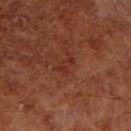{"biopsy_status": "not biopsied; imaged during a skin examination", "lesion_size": {"long_diameter_mm_approx": 2.5}, "site": "right lower leg", "lighting": "cross-polarized", "image": {"source": "total-body photography crop", "field_of_view_mm": 15}, "patient": {"sex": "male", "age_approx": 70}}